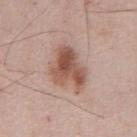{
  "biopsy_status": "not biopsied; imaged during a skin examination",
  "site": "abdomen",
  "lesion_size": {
    "long_diameter_mm_approx": 5.0
  },
  "patient": {
    "sex": "male",
    "age_approx": 55
  },
  "image": {
    "source": "total-body photography crop",
    "field_of_view_mm": 15
  },
  "lighting": "white-light"
}